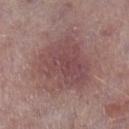* anatomic site · the leg
* lighting · white-light
* imaging modality · ~15 mm crop, total-body skin-cancer survey
* patient · male, roughly 75 years of age
* automated metrics · a mean CIELAB color near L≈48 a*≈21 b*≈19 and a lesion–skin lightness drop of about 8